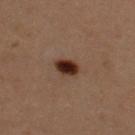Notes:
• follow-up: total-body-photography surveillance lesion; no biopsy